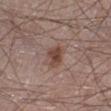Recorded during total-body skin imaging; not selected for excision or biopsy.
The lesion is on the leg.
Cropped from a whole-body photographic skin survey; the tile spans about 15 mm.
The lesion-visualizer software estimated an area of roughly 5.5 mm², an outline eccentricity of about 0.55 (0 = round, 1 = elongated), and a shape-asymmetry score of about 0.3 (0 = symmetric). The analysis additionally found a mean CIELAB color near L≈45 a*≈18 b*≈24 and a lesion-to-skin contrast of about 8.5 (normalized; higher = more distinct). It also reported peripheral color asymmetry of about 1. And it measured lesion-presence confidence of about 100/100.
Captured under white-light illumination.
Approximately 2.5 mm at its widest.
A male patient, aged 63–67.